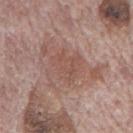Case summary:
- workup · catalogued during a skin exam; not biopsied
- diameter · ≈8 mm
- patient · male, in their 70s
- lighting · white-light
- location · the mid back
- acquisition · ~15 mm tile from a whole-body skin photo